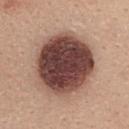Clinical impression:
This lesion was catalogued during total-body skin photography and was not selected for biopsy.
Background:
About 7.5 mm across. Cropped from a whole-body photographic skin survey; the tile spans about 15 mm. The subject is a female aged around 35. The total-body-photography lesion software estimated an area of roughly 42 mm². And it measured an automated nevus-likeness rating near 80 out of 100 and a detector confidence of about 100 out of 100 that the crop contains a lesion. The lesion is on the upper back. Captured under white-light illumination.